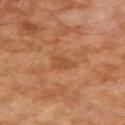Impression: No biopsy was performed on this lesion — it was imaged during a full skin examination and was not determined to be concerning. Image and clinical context: A male patient, aged around 70. A region of skin cropped from a whole-body photographic capture, roughly 15 mm wide. Located on the arm.